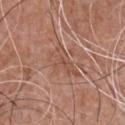The lesion was tiled from a total-body skin photograph and was not biopsied. A region of skin cropped from a whole-body photographic capture, roughly 15 mm wide. Approximately 4.5 mm at its widest. The subject is a male aged approximately 55. Located on the front of the torso.